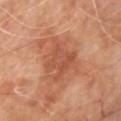<case>
  <biopsy_status>not biopsied; imaged during a skin examination</biopsy_status>
  <automated_metrics>
    <cielab_L>50</cielab_L>
    <cielab_a>26</cielab_a>
    <cielab_b>32</cielab_b>
    <vs_skin_darker_L>8.0</vs_skin_darker_L>
    <vs_skin_contrast_norm>6.0</vs_skin_contrast_norm>
    <border_irregularity_0_10>5.0</border_irregularity_0_10>
    <peripheral_color_asymmetry>1.0</peripheral_color_asymmetry>
  </automated_metrics>
  <site>front of the torso</site>
  <image>
    <source>total-body photography crop</source>
    <field_of_view_mm>15</field_of_view_mm>
  </image>
  <patient>
    <sex>male</sex>
    <age_approx>65</age_approx>
  </patient>
  <lighting>cross-polarized</lighting>
  <lesion_size>
    <long_diameter_mm_approx>5.5</long_diameter_mm_approx>
  </lesion_size>
</case>